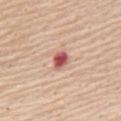Imaged during a routine full-body skin examination; the lesion was not biopsied and no histopathology is available. A region of skin cropped from a whole-body photographic capture, roughly 15 mm wide. A male patient, aged approximately 65. An algorithmic analysis of the crop reported roughly 16 lightness units darker than nearby skin and a lesion-to-skin contrast of about 10 (normalized; higher = more distinct). And it measured an automated nevus-likeness rating near 0 out of 100 and a lesion-detection confidence of about 100/100. Located on the chest.